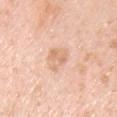This lesion was catalogued during total-body skin photography and was not selected for biopsy. The lesion is located on the chest. Longest diameter approximately 3.5 mm. The subject is a male about 50 years old. Cropped from a whole-body photographic skin survey; the tile spans about 15 mm. The total-body-photography lesion software estimated a lesion area of about 5.5 mm² and two-axis asymmetry of about 0.45. And it measured a border-irregularity index near 4.5/10, internal color variation of about 2 on a 0–10 scale, and peripheral color asymmetry of about 0.5. It also reported a nevus-likeness score of about 0/100 and lesion-presence confidence of about 100/100. Captured under white-light illumination.